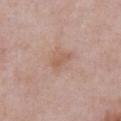Q: Was a biopsy performed?
A: imaged on a skin check; not biopsied
Q: What is the imaging modality?
A: ~15 mm tile from a whole-body skin photo
Q: Lesion location?
A: the abdomen
Q: Who is the patient?
A: male, aged approximately 55